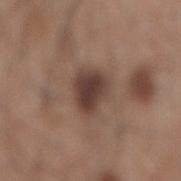A 15 mm crop from a total-body photograph taken for skin-cancer surveillance.
The recorded lesion diameter is about 4 mm.
The patient is a male aged 58–62.
From the mid back.